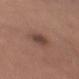No biopsy was performed on this lesion — it was imaged during a full skin examination and was not determined to be concerning.
The patient is a male in their mid-50s.
A 15 mm close-up extracted from a 3D total-body photography capture.
Located on the chest.
Longest diameter approximately 3 mm.
Captured under white-light illumination.